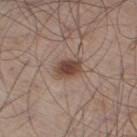Impression:
The lesion was tiled from a total-body skin photograph and was not biopsied.
Context:
A close-up tile cropped from a whole-body skin photograph, about 15 mm across. Measured at roughly 3.5 mm in maximum diameter. Automated tile analysis of the lesion measured a lesion area of about 6 mm², a shape eccentricity near 0.8, and two-axis asymmetry of about 0.15. And it measured a mean CIELAB color near L≈44 a*≈17 b*≈25 and a normalized lesion–skin contrast near 10. The analysis additionally found a nevus-likeness score of about 95/100 and lesion-presence confidence of about 100/100. The tile uses white-light illumination. A male patient aged approximately 60. On the right thigh.